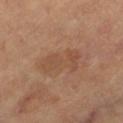<tbp_lesion>
  <biopsy_status>not biopsied; imaged during a skin examination</biopsy_status>
  <lesion_size>
    <long_diameter_mm_approx>5.0</long_diameter_mm_approx>
  </lesion_size>
  <patient>
    <age_approx>60</age_approx>
  </patient>
  <site>left thigh</site>
  <image>
    <source>total-body photography crop</source>
    <field_of_view_mm>15</field_of_view_mm>
  </image>
  <lighting>cross-polarized</lighting>
</tbp_lesion>